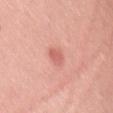<case>
<biopsy_status>not biopsied; imaged during a skin examination</biopsy_status>
<site>right thigh</site>
<lesion_size>
  <long_diameter_mm_approx>3.0</long_diameter_mm_approx>
</lesion_size>
<patient>
  <sex>female</sex>
  <age_approx>40</age_approx>
</patient>
<automated_metrics>
  <cielab_L>62</cielab_L>
  <cielab_a>30</cielab_a>
  <cielab_b>29</cielab_b>
  <vs_skin_darker_L>10.0</vs_skin_darker_L>
</automated_metrics>
<image>
  <source>total-body photography crop</source>
  <field_of_view_mm>15</field_of_view_mm>
</image>
</case>